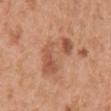The lesion was photographed on a routine skin check and not biopsied; there is no pathology result.
Automated tile analysis of the lesion measured a lesion area of about 13 mm², an eccentricity of roughly 0.9, and a symmetry-axis asymmetry near 0.55. The software also gave a mean CIELAB color near L≈55 a*≈24 b*≈33 and a lesion-to-skin contrast of about 6.5 (normalized; higher = more distinct).
A female patient aged 38 to 42.
The lesion is on the right upper arm.
Cropped from a total-body skin-imaging series; the visible field is about 15 mm.
Imaged with white-light lighting.
Measured at roughly 6.5 mm in maximum diameter.